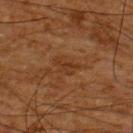Imaged during a routine full-body skin examination; the lesion was not biopsied and no histopathology is available. A male subject, aged approximately 65. A 15 mm close-up tile from a total-body photography series done for melanoma screening. Located on the upper back. Measured at roughly 3 mm in maximum diameter. The total-body-photography lesion software estimated internal color variation of about 0.5 on a 0–10 scale.